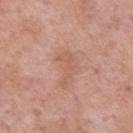Recorded during total-body skin imaging; not selected for excision or biopsy. A 15 mm close-up extracted from a 3D total-body photography capture. The lesion is on the left upper arm. A male patient about 55 years old.